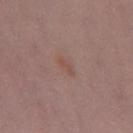biopsy status=catalogued during a skin exam; not biopsied
anatomic site=the left thigh
lighting=white-light illumination
acquisition=total-body-photography crop, ~15 mm field of view
patient=female, in their 30s
lesion size=~2.5 mm (longest diameter)